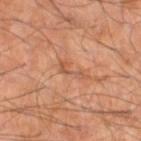| field | value |
|---|---|
| patient | male, aged 53 to 57 |
| anatomic site | the right thigh |
| image source | ~15 mm tile from a whole-body skin photo |